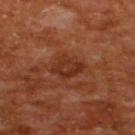<lesion>
  <biopsy_status>not biopsied; imaged during a skin examination</biopsy_status>
  <lesion_size>
    <long_diameter_mm_approx>4.0</long_diameter_mm_approx>
  </lesion_size>
  <image>
    <source>total-body photography crop</source>
    <field_of_view_mm>15</field_of_view_mm>
  </image>
  <patient>
    <sex>male</sex>
    <age_approx>65</age_approx>
  </patient>
  <site>upper back</site>
  <lighting>cross-polarized</lighting>
</lesion>